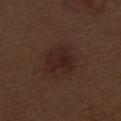| key | value |
|---|---|
| biopsy status | no biopsy performed (imaged during a skin exam) |
| body site | the leg |
| acquisition | ~15 mm tile from a whole-body skin photo |
| subject | male, aged 68 to 72 |